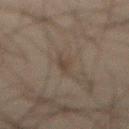- imaging modality · ~15 mm tile from a whole-body skin photo
- site · the abdomen
- illumination · cross-polarized illumination
- subject · male, aged 43–47